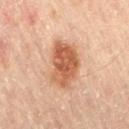A male patient, aged 43–47. About 5.5 mm across. A 15 mm close-up tile from a total-body photography series done for melanoma screening. The lesion is located on the right thigh. Captured under cross-polarized illumination.